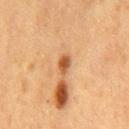Imaged during a routine full-body skin examination; the lesion was not biopsied and no histopathology is available. Cropped from a whole-body photographic skin survey; the tile spans about 15 mm. Approximately 2.5 mm at its widest. An algorithmic analysis of the crop reported an area of roughly 3.5 mm², an outline eccentricity of about 0.7 (0 = round, 1 = elongated), and a symmetry-axis asymmetry near 0.25. And it measured a border-irregularity index near 2/10, a color-variation rating of about 3.5/10, and radial color variation of about 1. It also reported a nevus-likeness score of about 100/100. The tile uses cross-polarized illumination. The lesion is on the mid back. A male patient, about 75 years old.